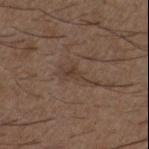This lesion was catalogued during total-body skin photography and was not selected for biopsy. Located on the chest. A male subject aged around 50. A roughly 15 mm field-of-view crop from a total-body skin photograph.